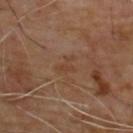Clinical impression:
Part of a total-body skin-imaging series; this lesion was reviewed on a skin check and was not flagged for biopsy.
Background:
A 15 mm close-up extracted from a 3D total-body photography capture. The subject is a male roughly 65 years of age. Captured under cross-polarized illumination. An algorithmic analysis of the crop reported a footprint of about 3 mm². The software also gave a lesion color around L≈39 a*≈18 b*≈29 in CIELAB and a normalized lesion–skin contrast near 5. And it measured border irregularity of about 7 on a 0–10 scale, a within-lesion color-variation index near 0/10, and a peripheral color-asymmetry measure near 0. From the upper back.